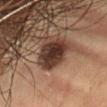Clinical impression: No biopsy was performed on this lesion — it was imaged during a full skin examination and was not determined to be concerning. Image and clinical context: A 15 mm close-up extracted from a 3D total-body photography capture. On the abdomen. The subject is a male approximately 55 years of age.